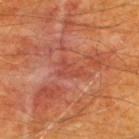Impression: The lesion was tiled from a total-body skin photograph and was not biopsied. Image and clinical context: The total-body-photography lesion software estimated a lesion color around L≈44 a*≈30 b*≈30 in CIELAB, roughly 6 lightness units darker than nearby skin, and a lesion-to-skin contrast of about 4.5 (normalized; higher = more distinct). The analysis additionally found peripheral color asymmetry of about 0. The analysis additionally found an automated nevus-likeness rating near 0 out of 100 and a lesion-detection confidence of about 85/100. A male subject aged around 60. Measured at roughly 3 mm in maximum diameter. On the upper back. This is a cross-polarized tile. A close-up tile cropped from a whole-body skin photograph, about 15 mm across.